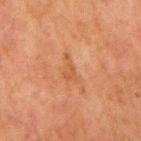Impression: The lesion was tiled from a total-body skin photograph and was not biopsied. Context: A 15 mm crop from a total-body photograph taken for skin-cancer surveillance. About 3.5 mm across. This is a cross-polarized tile. A male subject in their 80s. The lesion is on the mid back.